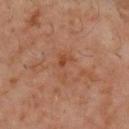Captured during whole-body skin photography for melanoma surveillance; the lesion was not biopsied. Located on the back. Automated tile analysis of the lesion measured a border-irregularity rating of about 7/10. It also reported a lesion-detection confidence of about 100/100. A male subject approximately 60 years of age. A 15 mm crop from a total-body photograph taken for skin-cancer surveillance.